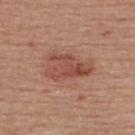A male patient aged 53 to 57. The recorded lesion diameter is about 5.5 mm. This image is a 15 mm lesion crop taken from a total-body photograph. The tile uses white-light illumination. On the upper back. An algorithmic analysis of the crop reported a peripheral color-asymmetry measure near 2. The analysis additionally found an automated nevus-likeness rating near 80 out of 100 and lesion-presence confidence of about 100/100.